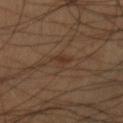{
  "biopsy_status": "not biopsied; imaged during a skin examination",
  "image": {
    "source": "total-body photography crop",
    "field_of_view_mm": 15
  },
  "patient": {
    "sex": "male",
    "age_approx": 40
  },
  "site": "right lower leg"
}